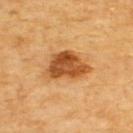| key | value |
|---|---|
| biopsy status | total-body-photography surveillance lesion; no biopsy |
| body site | the upper back |
| patient | male, roughly 60 years of age |
| automated metrics | an area of roughly 14 mm² and an eccentricity of roughly 0.7; an average lesion color of about L≈50 a*≈25 b*≈43 (CIELAB) and about 15 CIELAB-L* units darker than the surrounding skin |
| size | ≈5.5 mm |
| tile lighting | cross-polarized |
| image | ~15 mm tile from a whole-body skin photo |